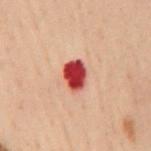The lesion was tiled from a total-body skin photograph and was not biopsied.
A male patient, aged 48 to 52.
The lesion is located on the mid back.
This image is a 15 mm lesion crop taken from a total-body photograph.